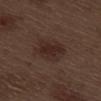Impression: Imaged during a routine full-body skin examination; the lesion was not biopsied and no histopathology is available. Clinical summary: The patient is a male aged approximately 70. From the right thigh. A 15 mm close-up tile from a total-body photography series done for melanoma screening. Approximately 4.5 mm at its widest. Imaged with white-light lighting.